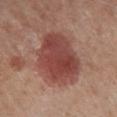Automated tile analysis of the lesion measured a lesion-to-skin contrast of about 9 (normalized; higher = more distinct).
The lesion is located on the right lower leg.
About 7 mm across.
Cropped from a total-body skin-imaging series; the visible field is about 15 mm.
A female patient about 55 years old.
Captured under cross-polarized illumination.